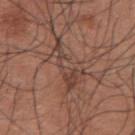This lesion was catalogued during total-body skin photography and was not selected for biopsy.
Located on the upper back.
The lesion's longest dimension is about 6.5 mm.
The subject is a male aged around 35.
A 15 mm crop from a total-body photograph taken for skin-cancer surveillance.